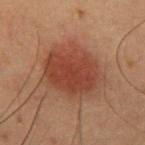Impression: This lesion was catalogued during total-body skin photography and was not selected for biopsy. Context: A lesion tile, about 15 mm wide, cut from a 3D total-body photograph. Longest diameter approximately 5.5 mm. A male subject, about 50 years old. From the abdomen.